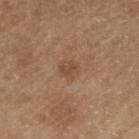This lesion was catalogued during total-body skin photography and was not selected for biopsy.
The subject is a male aged 68 to 72.
Located on the left thigh.
A roughly 15 mm field-of-view crop from a total-body skin photograph.
About 2.5 mm across.
Imaged with cross-polarized lighting.